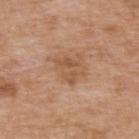follow-up: imaged on a skin check; not biopsied | body site: the upper back | TBP lesion metrics: a lesion area of about 6 mm², an eccentricity of roughly 0.7, and a symmetry-axis asymmetry near 0.45; border irregularity of about 5.5 on a 0–10 scale and peripheral color asymmetry of about 1.5 | imaging modality: 15 mm crop, total-body photography | tile lighting: white-light | patient: male, aged 58 to 62 | size: about 3.5 mm.